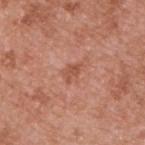Q: Lesion location?
A: the upper back
Q: What are the patient's age and sex?
A: male, aged 53–57
Q: What kind of image is this?
A: ~15 mm tile from a whole-body skin photo